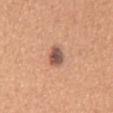notes — catalogued during a skin exam; not biopsied
TBP lesion metrics — a lesion color around L≈54 a*≈22 b*≈27 in CIELAB
size — ~3 mm (longest diameter)
patient — male, in their mid-50s
acquisition — ~15 mm tile from a whole-body skin photo
tile lighting — white-light
body site — the front of the torso